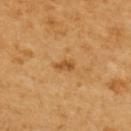Q: Is there a histopathology result?
A: imaged on a skin check; not biopsied